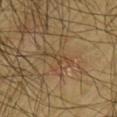Findings:
– notes · no biopsy performed (imaged during a skin exam)
– patient · male, aged 63 to 67
– lesion diameter · about 1.5 mm
– image · ~15 mm crop, total-body skin-cancer survey
– site · the arm